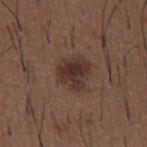tile lighting: white-light illumination
image source: ~15 mm crop, total-body skin-cancer survey
subject: male, in their 50s
location: the chest